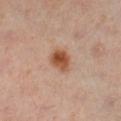Clinical impression: Recorded during total-body skin imaging; not selected for excision or biopsy. Image and clinical context: A female subject, approximately 40 years of age. A 15 mm crop from a total-body photograph taken for skin-cancer surveillance. Automated image analysis of the tile measured a footprint of about 6.5 mm² and a symmetry-axis asymmetry near 0.2. The software also gave an average lesion color of about L≈53 a*≈23 b*≈33 (CIELAB), about 13 CIELAB-L* units darker than the surrounding skin, and a normalized border contrast of about 10. It also reported a border-irregularity rating of about 2/10 and a peripheral color-asymmetry measure near 1.5. And it measured an automated nevus-likeness rating near 100 out of 100 and a lesion-detection confidence of about 100/100. Captured under cross-polarized illumination. Longest diameter approximately 3 mm. The lesion is located on the left leg.